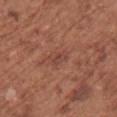Findings:
- notes — total-body-photography surveillance lesion; no biopsy
- site — the upper back
- illumination — white-light
- image source — ~15 mm tile from a whole-body skin photo
- subject — female, roughly 75 years of age